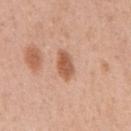The lesion's longest dimension is about 3.5 mm.
A male subject aged around 55.
This is a white-light tile.
On the chest.
Cropped from a total-body skin-imaging series; the visible field is about 15 mm.
The lesion-visualizer software estimated a mean CIELAB color near L≈57 a*≈24 b*≈33 and a normalized lesion–skin contrast near 8.5. It also reported border irregularity of about 2 on a 0–10 scale, internal color variation of about 3 on a 0–10 scale, and radial color variation of about 1. And it measured a detector confidence of about 100 out of 100 that the crop contains a lesion.